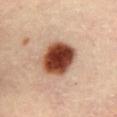Impression:
The lesion was photographed on a routine skin check and not biopsied; there is no pathology result.